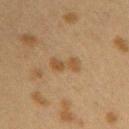biopsy_status: not biopsied; imaged during a skin examination
patient:
  sex: female
  age_approx: 40
lesion_size:
  long_diameter_mm_approx: 3.5
image:
  source: total-body photography crop
  field_of_view_mm: 15
site: arm